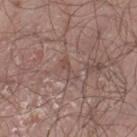Impression: Recorded during total-body skin imaging; not selected for excision or biopsy. Context: The tile uses white-light illumination. A male patient, roughly 55 years of age. Cropped from a total-body skin-imaging series; the visible field is about 15 mm. On the left thigh. Longest diameter approximately 3 mm. Automated tile analysis of the lesion measured a footprint of about 2.5 mm², an eccentricity of roughly 0.95, and a shape-asymmetry score of about 0.45 (0 = symmetric). The software also gave roughly 6 lightness units darker than nearby skin and a normalized border contrast of about 4.5. It also reported a classifier nevus-likeness of about 0/100 and lesion-presence confidence of about 70/100.